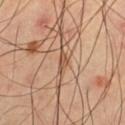workup: imaged on a skin check; not biopsied | site: the left thigh | subject: male, aged 63–67 | image source: ~15 mm tile from a whole-body skin photo.